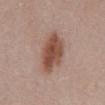The lesion was tiled from a total-body skin photograph and was not biopsied.
This image is a 15 mm lesion crop taken from a total-body photograph.
The lesion-visualizer software estimated a mean CIELAB color near L≈49 a*≈21 b*≈27 and about 13 CIELAB-L* units darker than the surrounding skin. The analysis additionally found an automated nevus-likeness rating near 100 out of 100.
A male subject, in their mid- to late 50s.
From the mid back.
Imaged with white-light lighting.